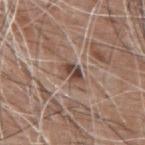Approximately 2.5 mm at its widest. A male patient, in their 60s. The lesion-visualizer software estimated a footprint of about 4 mm², an outline eccentricity of about 0.75 (0 = round, 1 = elongated), and a symmetry-axis asymmetry near 0.25. The software also gave a lesion–skin lightness drop of about 13. The software also gave lesion-presence confidence of about 70/100. A lesion tile, about 15 mm wide, cut from a 3D total-body photograph. On the upper back. Imaged with white-light lighting.